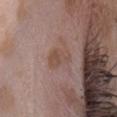patient — female, in their mid- to late 20s | site — the head or neck | acquisition — 15 mm crop, total-body photography.